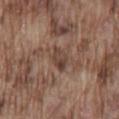Assessment: Imaged during a routine full-body skin examination; the lesion was not biopsied and no histopathology is available. Clinical summary: Imaged with white-light lighting. Longest diameter approximately 3 mm. A male patient, approximately 75 years of age. On the lower back. A 15 mm crop from a total-body photograph taken for skin-cancer surveillance.